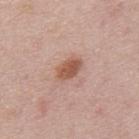Case summary:
– notes · catalogued during a skin exam; not biopsied
– patient · male, approximately 45 years of age
– body site · the mid back
– automated lesion analysis · a lesion area of about 5.5 mm², a shape eccentricity near 0.8, and a symmetry-axis asymmetry near 0.25; a lesion color around L≈55 a*≈22 b*≈29 in CIELAB and roughly 12 lightness units darker than nearby skin; a border-irregularity index near 2.5/10 and a within-lesion color-variation index near 2.5/10
– acquisition · ~15 mm tile from a whole-body skin photo
– lesion diameter · ~3.5 mm (longest diameter)
– lighting · white-light illumination